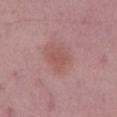lighting: white-light | image-analysis metrics: a shape-asymmetry score of about 0.3 (0 = symmetric); an average lesion color of about L≈52 a*≈25 b*≈23 (CIELAB), roughly 6 lightness units darker than nearby skin, and a normalized lesion–skin contrast near 5; a border-irregularity index near 3/10, a color-variation rating of about 1.5/10, and a peripheral color-asymmetry measure near 0.5; lesion-presence confidence of about 100/100 | subject: male, in their mid- to late 50s | site: the abdomen | imaging modality: ~15 mm tile from a whole-body skin photo.